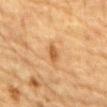The lesion was tiled from a total-body skin photograph and was not biopsied. About 3 mm across. The lesion is on the abdomen. The tile uses cross-polarized illumination. Cropped from a total-body skin-imaging series; the visible field is about 15 mm. An algorithmic analysis of the crop reported roughly 9 lightness units darker than nearby skin. The analysis additionally found a within-lesion color-variation index near 2/10 and peripheral color asymmetry of about 0.5. The subject is a male roughly 85 years of age or older.